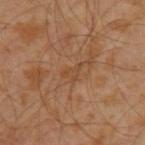| feature | finding |
|---|---|
| biopsy status | total-body-photography surveillance lesion; no biopsy |
| automated metrics | a lesion area of about 3 mm², an outline eccentricity of about 0.8 (0 = round, 1 = elongated), and a symmetry-axis asymmetry near 0.5; a mean CIELAB color near L≈45 a*≈19 b*≈33, a lesion–skin lightness drop of about 6, and a lesion-to-skin contrast of about 5 (normalized; higher = more distinct) |
| patient | male, about 30 years old |
| imaging modality | total-body-photography crop, ~15 mm field of view |
| site | the left upper arm |